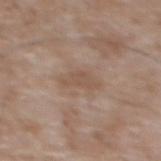notes: total-body-photography surveillance lesion; no biopsy | TBP lesion metrics: an average lesion color of about L≈53 a*≈16 b*≈27 (CIELAB), a lesion–skin lightness drop of about 6, and a normalized lesion–skin contrast near 5; a border-irregularity rating of about 4/10, internal color variation of about 1 on a 0–10 scale, and a peripheral color-asymmetry measure near 0.5 | diameter: about 4 mm | location: the mid back | patient: male, in their 50s | imaging modality: 15 mm crop, total-body photography.